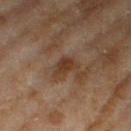The total-body-photography lesion software estimated an outline eccentricity of about 0.85 (0 = round, 1 = elongated) and a shape-asymmetry score of about 0.4 (0 = symmetric). It also reported a classifier nevus-likeness of about 0/100 and a lesion-detection confidence of about 95/100. Measured at roughly 3.5 mm in maximum diameter. The patient is a female in their 60s. A lesion tile, about 15 mm wide, cut from a 3D total-body photograph. This is a cross-polarized tile. Located on the right thigh.